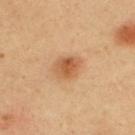Captured under cross-polarized illumination. A male subject, aged approximately 40. From the back. About 3.5 mm across. A 15 mm close-up extracted from a 3D total-body photography capture.